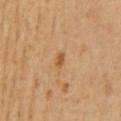Q: Was this lesion biopsied?
A: imaged on a skin check; not biopsied
Q: Patient demographics?
A: female, approximately 55 years of age
Q: What kind of image is this?
A: 15 mm crop, total-body photography
Q: Where on the body is the lesion?
A: the mid back